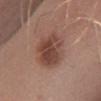{
  "biopsy_status": "not biopsied; imaged during a skin examination",
  "automated_metrics": {
    "color_variation_0_10": 4.5,
    "peripheral_color_asymmetry": 1.5,
    "nevus_likeness_0_100": 85,
    "lesion_detection_confidence_0_100": 100
  },
  "patient": {
    "sex": "male",
    "age_approx": 45
  },
  "lesion_size": {
    "long_diameter_mm_approx": 5.0
  },
  "site": "right lower leg",
  "lighting": "white-light",
  "image": {
    "source": "total-body photography crop",
    "field_of_view_mm": 15
  }
}